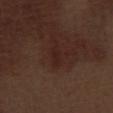Q: Was this lesion biopsied?
A: imaged on a skin check; not biopsied
Q: Lesion location?
A: the left thigh
Q: Illumination type?
A: white-light illumination
Q: What is the imaging modality?
A: total-body-photography crop, ~15 mm field of view
Q: What is the lesion's diameter?
A: ~2.5 mm (longest diameter)
Q: What are the patient's age and sex?
A: male, approximately 70 years of age